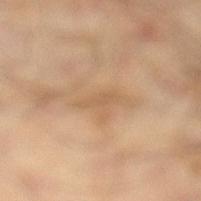Case summary:
• follow-up: imaged on a skin check; not biopsied
• body site: the left lower leg
• diameter: ~3 mm (longest diameter)
• illumination: cross-polarized illumination
• patient: male, approximately 65 years of age
• image source: ~15 mm crop, total-body skin-cancer survey